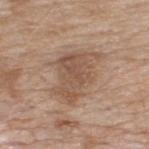A male subject, aged 63 to 67. From the upper back. This image is a 15 mm lesion crop taken from a total-body photograph.